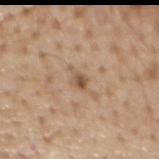Clinical impression:
Imaged during a routine full-body skin examination; the lesion was not biopsied and no histopathology is available.
Context:
Measured at roughly 2.5 mm in maximum diameter. A male patient, about 70 years old. This is a white-light tile. Cropped from a whole-body photographic skin survey; the tile spans about 15 mm. Located on the chest.